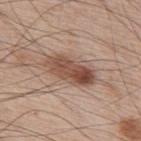Assessment:
Part of a total-body skin-imaging series; this lesion was reviewed on a skin check and was not flagged for biopsy.
Image and clinical context:
On the upper back. Imaged with white-light lighting. The recorded lesion diameter is about 6 mm. A male patient aged around 65. Cropped from a whole-body photographic skin survey; the tile spans about 15 mm.